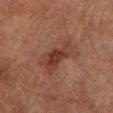Clinical impression: This lesion was catalogued during total-body skin photography and was not selected for biopsy. Acquisition and patient details: On the left lower leg. A 15 mm crop from a total-body photograph taken for skin-cancer surveillance. The subject is a male aged approximately 75.